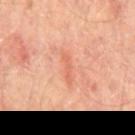Clinical impression:
Imaged during a routine full-body skin examination; the lesion was not biopsied and no histopathology is available.
Background:
This image is a 15 mm lesion crop taken from a total-body photograph. The lesion is located on the mid back. Imaged with cross-polarized lighting. Approximately 4 mm at its widest. A male patient aged around 65.